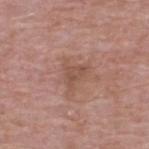The lesion was photographed on a routine skin check and not biopsied; there is no pathology result. The lesion is on the back. A close-up tile cropped from a whole-body skin photograph, about 15 mm across. Automated image analysis of the tile measured an area of roughly 3.5 mm², an eccentricity of roughly 0.7, and a shape-asymmetry score of about 0.55 (0 = symmetric). And it measured a border-irregularity index near 5/10, a within-lesion color-variation index near 2/10, and peripheral color asymmetry of about 0.5. A male subject, roughly 65 years of age. Captured under white-light illumination.